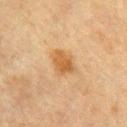biopsy_status: not biopsied; imaged during a skin examination
image:
  source: total-body photography crop
  field_of_view_mm: 15
site: chest
lesion_size:
  long_diameter_mm_approx: 3.0
patient:
  sex: female
  age_approx: 55